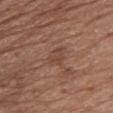| feature | finding |
|---|---|
| lesion diameter | about 2.5 mm |
| automated metrics | a border-irregularity rating of about 4/10, a within-lesion color-variation index near 2/10, and peripheral color asymmetry of about 0.5; a nevus-likeness score of about 0/100 |
| image source | total-body-photography crop, ~15 mm field of view |
| subject | female, in their mid- to late 70s |
| location | the chest |
| tile lighting | white-light |